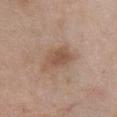Captured during whole-body skin photography for melanoma surveillance; the lesion was not biopsied. This is a white-light tile. Measured at roughly 5 mm in maximum diameter. Located on the front of the torso. A 15 mm crop from a total-body photograph taken for skin-cancer surveillance. A female subject in their 60s.